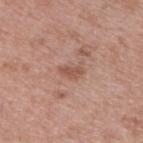follow-up — total-body-photography surveillance lesion; no biopsy | acquisition — ~15 mm tile from a whole-body skin photo | illumination — white-light | patient — male, in their 40s | image-analysis metrics — a lesion area of about 3 mm² and a symmetry-axis asymmetry near 0.35; internal color variation of about 1 on a 0–10 scale and radial color variation of about 0.5 | location — the back.